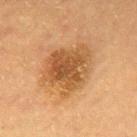| field | value |
|---|---|
| notes | no biopsy performed (imaged during a skin exam) |
| body site | the mid back |
| lighting | cross-polarized illumination |
| size | ~6.5 mm (longest diameter) |
| subject | female, aged around 60 |
| image | total-body-photography crop, ~15 mm field of view |